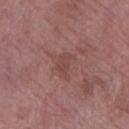– notes · no biopsy performed (imaged during a skin exam)
– image source · 15 mm crop, total-body photography
– subject · female, approximately 70 years of age
– image-analysis metrics · a footprint of about 4 mm², an eccentricity of roughly 0.85, and a symmetry-axis asymmetry near 0.45; a classifier nevus-likeness of about 0/100 and lesion-presence confidence of about 100/100
– anatomic site · the left thigh